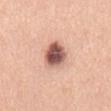The lesion was photographed on a routine skin check and not biopsied; there is no pathology result. The lesion is located on the mid back. Imaged with white-light lighting. The recorded lesion diameter is about 3.5 mm. The subject is a male aged 48–52. Automated tile analysis of the lesion measured a border-irregularity index near 2/10 and a peripheral color-asymmetry measure near 2. The software also gave a nevus-likeness score of about 60/100 and a lesion-detection confidence of about 100/100. A 15 mm close-up tile from a total-body photography series done for melanoma screening.